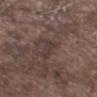This lesion was catalogued during total-body skin photography and was not selected for biopsy. A region of skin cropped from a whole-body photographic capture, roughly 15 mm wide. Located on the left thigh. This is a white-light tile. A male subject, approximately 75 years of age. The lesion's longest dimension is about 3 mm.